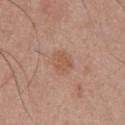| feature | finding |
|---|---|
| follow-up | total-body-photography surveillance lesion; no biopsy |
| imaging modality | ~15 mm tile from a whole-body skin photo |
| lighting | white-light |
| site | the chest |
| subject | male, aged around 55 |
| lesion size | ~3 mm (longest diameter) |